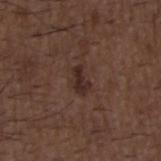<lesion>
  <biopsy_status>not biopsied; imaged during a skin examination</biopsy_status>
  <lesion_size>
    <long_diameter_mm_approx>3.5</long_diameter_mm_approx>
  </lesion_size>
  <image>
    <source>total-body photography crop</source>
    <field_of_view_mm>15</field_of_view_mm>
  </image>
  <patient>
    <sex>male</sex>
    <age_approx>50</age_approx>
  </patient>
  <site>upper back</site>
</lesion>